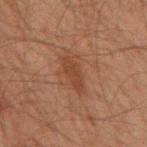  biopsy_status: not biopsied; imaged during a skin examination
  image:
    source: total-body photography crop
    field_of_view_mm: 15
  site: mid back
  lighting: cross-polarized
  lesion_size:
    long_diameter_mm_approx: 4.0
  patient:
    sex: male
    age_approx: 60